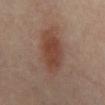Imaged during a routine full-body skin examination; the lesion was not biopsied and no histopathology is available. Imaged with cross-polarized lighting. Automated tile analysis of the lesion measured a border-irregularity rating of about 3/10, internal color variation of about 4 on a 0–10 scale, and radial color variation of about 1. The software also gave a classifier nevus-likeness of about 100/100 and lesion-presence confidence of about 100/100. A 15 mm close-up extracted from a 3D total-body photography capture. About 8 mm across. A female subject, aged approximately 50. The lesion is on the mid back.